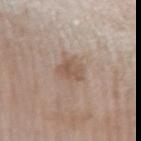Part of a total-body skin-imaging series; this lesion was reviewed on a skin check and was not flagged for biopsy. A female patient, roughly 75 years of age. Located on the left forearm. A region of skin cropped from a whole-body photographic capture, roughly 15 mm wide.